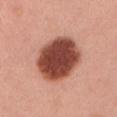Clinical impression:
No biopsy was performed on this lesion — it was imaged during a full skin examination and was not determined to be concerning.
Context:
The lesion is on the arm. This is a white-light tile. A lesion tile, about 15 mm wide, cut from a 3D total-body photograph. The lesion-visualizer software estimated a footprint of about 26 mm², a shape eccentricity near 0.55, and a shape-asymmetry score of about 0.1 (0 = symmetric). It also reported a mean CIELAB color near L≈46 a*≈27 b*≈29, roughly 22 lightness units darker than nearby skin, and a lesion-to-skin contrast of about 14.5 (normalized; higher = more distinct). The software also gave a border-irregularity index near 1/10, a within-lesion color-variation index near 4.5/10, and peripheral color asymmetry of about 1. It also reported lesion-presence confidence of about 100/100. The patient is a male aged around 60. Longest diameter approximately 6 mm.